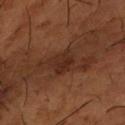<record>
<image>
  <source>total-body photography crop</source>
  <field_of_view_mm>15</field_of_view_mm>
</image>
<lesion_size>
  <long_diameter_mm_approx>3.0</long_diameter_mm_approx>
</lesion_size>
<automated_metrics>
  <area_mm2_approx>7.0</area_mm2_approx>
  <shape_asymmetry>0.3</shape_asymmetry>
</automated_metrics>
<lighting>cross-polarized</lighting>
<patient>
  <sex>male</sex>
  <age_approx>65</age_approx>
</patient>
<site>left forearm</site>
</record>